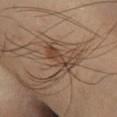About 4 mm across.
The lesion-visualizer software estimated a mean CIELAB color near L≈43 a*≈17 b*≈28, roughly 9 lightness units darker than nearby skin, and a normalized lesion–skin contrast near 7.5. And it measured a border-irregularity rating of about 5/10 and a within-lesion color-variation index near 3/10. The analysis additionally found a classifier nevus-likeness of about 90/100 and lesion-presence confidence of about 100/100.
This image is a 15 mm lesion crop taken from a total-body photograph.
A male patient roughly 55 years of age.
The lesion is on the left lower leg.